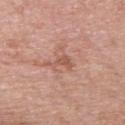subject=male, aged 53 to 57 | site=the front of the torso | imaging modality=~15 mm crop, total-body skin-cancer survey | lesion size=≈3.5 mm | TBP lesion metrics=a mean CIELAB color near L≈56 a*≈23 b*≈29, about 8 CIELAB-L* units darker than the surrounding skin, and a normalized border contrast of about 6; a classifier nevus-likeness of about 0/100 and a detector confidence of about 100 out of 100 that the crop contains a lesion | tile lighting=white-light illumination.